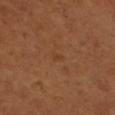Q: Is there a histopathology result?
A: total-body-photography surveillance lesion; no biopsy
Q: How was this image acquired?
A: ~15 mm tile from a whole-body skin photo
Q: Where on the body is the lesion?
A: the left forearm
Q: What are the patient's age and sex?
A: female, aged 53 to 57
Q: What lighting was used for the tile?
A: cross-polarized illumination